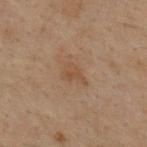workup: imaged on a skin check; not biopsied
lesion size: ~3 mm (longest diameter)
anatomic site: the upper back
image source: ~15 mm tile from a whole-body skin photo
subject: male, about 30 years old
automated lesion analysis: a lesion area of about 5 mm², an outline eccentricity of about 0.65 (0 = round, 1 = elongated), and a shape-asymmetry score of about 0.3 (0 = symmetric); a lesion color around L≈39 a*≈14 b*≈26 in CIELAB, a lesion–skin lightness drop of about 5, and a lesion-to-skin contrast of about 5 (normalized; higher = more distinct)
tile lighting: cross-polarized illumination